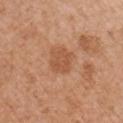Notes:
* biopsy status · total-body-photography surveillance lesion; no biopsy
* tile lighting · white-light illumination
* imaging modality · ~15 mm crop, total-body skin-cancer survey
* patient · female, about 40 years old
* size · ~3 mm (longest diameter)
* location · the front of the torso
* TBP lesion metrics · a color-variation rating of about 2/10 and peripheral color asymmetry of about 1